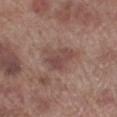Q: Was this lesion biopsied?
A: no biopsy performed (imaged during a skin exam)
Q: What is the anatomic site?
A: the left lower leg
Q: What kind of image is this?
A: ~15 mm tile from a whole-body skin photo
Q: Automated lesion metrics?
A: a footprint of about 9.5 mm², a shape eccentricity near 0.7, and a shape-asymmetry score of about 0.35 (0 = symmetric)
Q: What lighting was used for the tile?
A: white-light
Q: Patient demographics?
A: male, aged 68–72